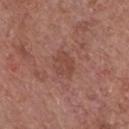<record>
<biopsy_status>not biopsied; imaged during a skin examination</biopsy_status>
<image>
  <source>total-body photography crop</source>
  <field_of_view_mm>15</field_of_view_mm>
</image>
<lighting>white-light</lighting>
<site>chest</site>
<automated_metrics>
  <area_mm2_approx>6.0</area_mm2_approx>
  <eccentricity>0.7</eccentricity>
  <vs_skin_contrast_norm>5.0</vs_skin_contrast_norm>
  <lesion_detection_confidence_0_100>100</lesion_detection_confidence_0_100>
</automated_metrics>
<lesion_size>
  <long_diameter_mm_approx>3.5</long_diameter_mm_approx>
</lesion_size>
<patient>
  <sex>male</sex>
  <age_approx>55</age_approx>
</patient>
</record>